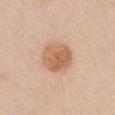Impression: Recorded during total-body skin imaging; not selected for excision or biopsy. Background: A male subject about 35 years old. Located on the left upper arm. A region of skin cropped from a whole-body photographic capture, roughly 15 mm wide.